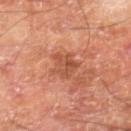Q: Was a biopsy performed?
A: imaged on a skin check; not biopsied
Q: What are the patient's age and sex?
A: male, aged approximately 70
Q: Lesion location?
A: the right lower leg
Q: How was the tile lit?
A: cross-polarized
Q: What did automated image analysis measure?
A: a shape eccentricity near 0.7 and a symmetry-axis asymmetry near 0.35; a mean CIELAB color near L≈51 a*≈28 b*≈33 and roughly 8 lightness units darker than nearby skin; border irregularity of about 4 on a 0–10 scale, a color-variation rating of about 4.5/10, and a peripheral color-asymmetry measure near 1.5; an automated nevus-likeness rating near 0 out of 100 and lesion-presence confidence of about 100/100
Q: What is the lesion's diameter?
A: ≈3.5 mm
Q: What kind of image is this?
A: 15 mm crop, total-body photography